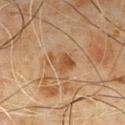Q: Was this lesion biopsied?
A: no biopsy performed (imaged during a skin exam)
Q: Patient demographics?
A: male, aged 58 to 62
Q: What did automated image analysis measure?
A: border irregularity of about 3.5 on a 0–10 scale, a color-variation rating of about 4/10, and a peripheral color-asymmetry measure near 1.5; a classifier nevus-likeness of about 20/100 and a lesion-detection confidence of about 100/100
Q: What is the lesion's diameter?
A: ~3.5 mm (longest diameter)
Q: What is the imaging modality?
A: 15 mm crop, total-body photography
Q: What is the anatomic site?
A: the chest
Q: How was the tile lit?
A: cross-polarized illumination